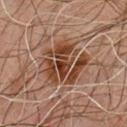Part of a total-body skin-imaging series; this lesion was reviewed on a skin check and was not flagged for biopsy.
Located on the chest.
Captured under cross-polarized illumination.
Cropped from a whole-body photographic skin survey; the tile spans about 15 mm.
A male patient roughly 45 years of age.